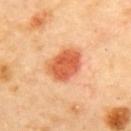The lesion was tiled from a total-body skin photograph and was not biopsied. Located on the back. A female patient roughly 60 years of age. The total-body-photography lesion software estimated a footprint of about 12 mm², a shape eccentricity near 0.7, and a shape-asymmetry score of about 0.2 (0 = symmetric). And it measured a mean CIELAB color near L≈63 a*≈34 b*≈43, roughly 15 lightness units darker than nearby skin, and a normalized lesion–skin contrast near 9. It also reported a border-irregularity rating of about 2/10, internal color variation of about 4 on a 0–10 scale, and radial color variation of about 1.5. The software also gave a nevus-likeness score of about 100/100. A 15 mm close-up tile from a total-body photography series done for melanoma screening. The lesion's longest dimension is about 4.5 mm.